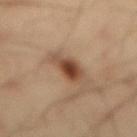Recorded during total-body skin imaging; not selected for excision or biopsy. The tile uses cross-polarized illumination. A 15 mm close-up extracted from a 3D total-body photography capture. About 4 mm across. The lesion is located on the mid back. A male subject aged 38–42.